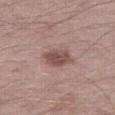The lesion was photographed on a routine skin check and not biopsied; there is no pathology result. The lesion is on the right thigh. A 15 mm close-up extracted from a 3D total-body photography capture. A male patient in their 40s. The total-body-photography lesion software estimated a border-irregularity rating of about 2/10, internal color variation of about 3 on a 0–10 scale, and peripheral color asymmetry of about 1. The analysis additionally found a nevus-likeness score of about 45/100. Captured under white-light illumination. The lesion's longest dimension is about 3.5 mm.